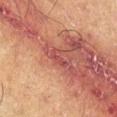| feature | finding |
|---|---|
| follow-up | total-body-photography surveillance lesion; no biopsy |
| acquisition | total-body-photography crop, ~15 mm field of view |
| body site | the leg |
| subject | male, about 60 years old |